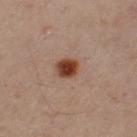* notes — total-body-photography surveillance lesion; no biopsy
* acquisition — 15 mm crop, total-body photography
* lighting — cross-polarized illumination
* automated lesion analysis — an outline eccentricity of about 0.45 (0 = round, 1 = elongated) and two-axis asymmetry of about 0.25; an average lesion color of about L≈44 a*≈16 b*≈24 (CIELAB), about 7 CIELAB-L* units darker than the surrounding skin, and a lesion-to-skin contrast of about 6.5 (normalized; higher = more distinct); a classifier nevus-likeness of about 100/100
* subject — male, aged approximately 50
* lesion size — ~4.5 mm (longest diameter)
* site — the left upper arm